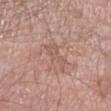Clinical impression:
The lesion was tiled from a total-body skin photograph and was not biopsied.
Context:
A male patient roughly 55 years of age. Imaged with white-light lighting. A roughly 15 mm field-of-view crop from a total-body skin photograph. The lesion is located on the left forearm.